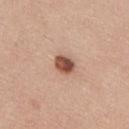workup=imaged on a skin check; not biopsied
subject=male, about 25 years old
acquisition=total-body-photography crop, ~15 mm field of view
lesion size=about 2.5 mm
location=the upper back
image-analysis metrics=a lesion–skin lightness drop of about 18
illumination=white-light illumination